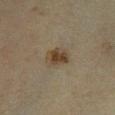Clinical impression: Imaged during a routine full-body skin examination; the lesion was not biopsied and no histopathology is available. Image and clinical context: Longest diameter approximately 3 mm. Cropped from a total-body skin-imaging series; the visible field is about 15 mm. A female patient in their 40s. The lesion-visualizer software estimated a lesion area of about 7 mm² and a symmetry-axis asymmetry near 0.2. The analysis additionally found border irregularity of about 2 on a 0–10 scale and peripheral color asymmetry of about 1.5. On the right lower leg.